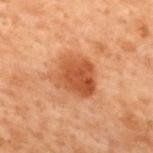Q: Is there a histopathology result?
A: no biopsy performed (imaged during a skin exam)
Q: Lesion location?
A: the back
Q: How was the tile lit?
A: cross-polarized
Q: Lesion size?
A: ~5 mm (longest diameter)
Q: Patient demographics?
A: male, in their 70s
Q: What is the imaging modality?
A: 15 mm crop, total-body photography
Q: Automated lesion metrics?
A: an outline eccentricity of about 0.55 (0 = round, 1 = elongated) and a symmetry-axis asymmetry near 0.25; a lesion color around L≈41 a*≈23 b*≈32 in CIELAB, a lesion–skin lightness drop of about 10, and a normalized lesion–skin contrast near 8; a nevus-likeness score of about 90/100 and a detector confidence of about 100 out of 100 that the crop contains a lesion